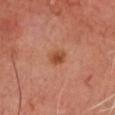Recorded during total-body skin imaging; not selected for excision or biopsy. About 2.5 mm across. The lesion is located on the head or neck. A male patient aged 68–72. Captured under cross-polarized illumination. A region of skin cropped from a whole-body photographic capture, roughly 15 mm wide.